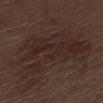Assessment: Recorded during total-body skin imaging; not selected for excision or biopsy. Image and clinical context: A 15 mm close-up extracted from a 3D total-body photography capture. Imaged with white-light lighting. On the left thigh. The recorded lesion diameter is about 11 mm. The patient is a male in their 70s.